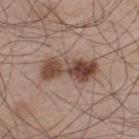Findings:
– patient: male, aged 43–47
– acquisition: ~15 mm tile from a whole-body skin photo
– site: the leg
– lesion diameter: ≈6.5 mm
– lighting: white-light illumination